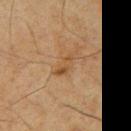This lesion was catalogued during total-body skin photography and was not selected for biopsy. A 15 mm crop from a total-body photograph taken for skin-cancer surveillance. Imaged with cross-polarized lighting. From the chest. Approximately 3 mm at its widest. A male subject, about 65 years old. The lesion-visualizer software estimated an outline eccentricity of about 0.9 (0 = round, 1 = elongated) and a symmetry-axis asymmetry near 0.35. It also reported border irregularity of about 3.5 on a 0–10 scale and a peripheral color-asymmetry measure near 1.5.